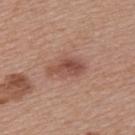This lesion was catalogued during total-body skin photography and was not selected for biopsy.
A female subject aged approximately 45.
Longest diameter approximately 4.5 mm.
The tile uses white-light illumination.
Located on the left upper arm.
A 15 mm crop from a total-body photograph taken for skin-cancer surveillance.